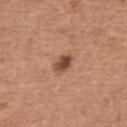image-analysis metrics: an average lesion color of about L≈47 a*≈23 b*≈29 (CIELAB), roughly 14 lightness units darker than nearby skin, and a lesion-to-skin contrast of about 10 (normalized; higher = more distinct); a border-irregularity index near 2/10 and peripheral color asymmetry of about 1 | site: the abdomen | diameter: ~2.5 mm (longest diameter) | imaging modality: 15 mm crop, total-body photography | tile lighting: white-light | subject: male, in their mid- to late 60s.